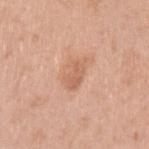Q: What did automated image analysis measure?
A: an area of roughly 6 mm², a shape eccentricity near 0.8, and two-axis asymmetry of about 0.35; a mean CIELAB color near L≈62 a*≈23 b*≈33, about 8 CIELAB-L* units darker than the surrounding skin, and a normalized lesion–skin contrast near 5.5; border irregularity of about 4.5 on a 0–10 scale, a within-lesion color-variation index near 1.5/10, and peripheral color asymmetry of about 0.5
Q: Lesion location?
A: the left upper arm
Q: Patient demographics?
A: female, roughly 40 years of age
Q: What is the lesion's diameter?
A: ~3.5 mm (longest diameter)
Q: What is the imaging modality?
A: 15 mm crop, total-body photography